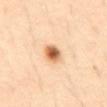Recorded during total-body skin imaging; not selected for excision or biopsy. A male subject, in their 40s. Located on the abdomen. A roughly 15 mm field-of-view crop from a total-body skin photograph. About 2.5 mm across. Imaged with cross-polarized lighting.